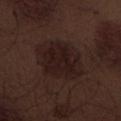{"biopsy_status": "not biopsied; imaged during a skin examination", "automated_metrics": {"cielab_L": 18, "cielab_a": 15, "cielab_b": 16, "vs_skin_darker_L": 6.0, "vs_skin_contrast_norm": 8.5, "border_irregularity_0_10": 1.5, "color_variation_0_10": 3.0, "peripheral_color_asymmetry": 1.0}, "site": "front of the torso", "patient": {"sex": "male", "age_approx": 70}, "image": {"source": "total-body photography crop", "field_of_view_mm": 15}, "lesion_size": {"long_diameter_mm_approx": 6.5}, "lighting": "white-light"}